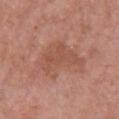biopsy status = catalogued during a skin exam; not biopsied
size = ≈6 mm
patient = female, in their 60s
acquisition = 15 mm crop, total-body photography
location = the chest